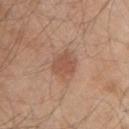<record>
  <biopsy_status>not biopsied; imaged during a skin examination</biopsy_status>
  <automated_metrics>
    <area_mm2_approx>7.0</area_mm2_approx>
    <eccentricity>0.45</eccentricity>
    <shape_asymmetry>0.25</shape_asymmetry>
    <nevus_likeness_0_100>70</nevus_likeness_0_100>
    <lesion_detection_confidence_0_100>100</lesion_detection_confidence_0_100>
  </automated_metrics>
  <lighting>white-light</lighting>
  <image>
    <source>total-body photography crop</source>
    <field_of_view_mm>15</field_of_view_mm>
  </image>
  <patient>
    <sex>male</sex>
    <age_approx>45</age_approx>
  </patient>
  <site>right upper arm</site>
  <lesion_size>
    <long_diameter_mm_approx>3.0</long_diameter_mm_approx>
  </lesion_size>
</record>